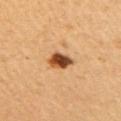notes: total-body-photography surveillance lesion; no biopsy
lighting: cross-polarized
size: ~3 mm (longest diameter)
imaging modality: ~15 mm tile from a whole-body skin photo
image-analysis metrics: a footprint of about 5.5 mm², an eccentricity of roughly 0.6, and a shape-asymmetry score of about 0.2 (0 = symmetric); a mean CIELAB color near L≈48 a*≈25 b*≈39 and about 20 CIELAB-L* units darker than the surrounding skin; a border-irregularity index near 1.5/10 and radial color variation of about 2; a nevus-likeness score of about 100/100 and a detector confidence of about 100 out of 100 that the crop contains a lesion
location: the right upper arm
patient: male, about 60 years old